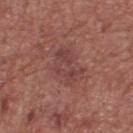{
  "biopsy_status": "not biopsied; imaged during a skin examination",
  "patient": {
    "sex": "male",
    "age_approx": 75
  },
  "lighting": "white-light",
  "site": "upper back",
  "image": {
    "source": "total-body photography crop",
    "field_of_view_mm": 15
  }
}